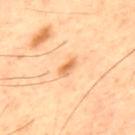biopsy status=catalogued during a skin exam; not biopsied